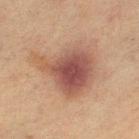Impression:
Captured during whole-body skin photography for melanoma surveillance; the lesion was not biopsied.
Acquisition and patient details:
Approximately 7 mm at its widest. The patient is a female approximately 55 years of age. The tile uses cross-polarized illumination. A lesion tile, about 15 mm wide, cut from a 3D total-body photograph. Located on the left thigh.